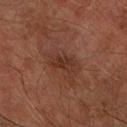Part of a total-body skin-imaging series; this lesion was reviewed on a skin check and was not flagged for biopsy.
A region of skin cropped from a whole-body photographic capture, roughly 15 mm wide.
The recorded lesion diameter is about 3 mm.
From the right forearm.
A male subject in their mid-70s.